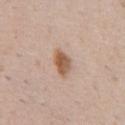The lesion was photographed on a routine skin check and not biopsied; there is no pathology result.